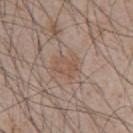Acquisition and patient details:
Located on the chest. Approximately 3 mm at its widest. Cropped from a total-body skin-imaging series; the visible field is about 15 mm. A male subject, roughly 45 years of age. The total-body-photography lesion software estimated an average lesion color of about L≈52 a*≈17 b*≈27 (CIELAB), about 6 CIELAB-L* units darker than the surrounding skin, and a lesion-to-skin contrast of about 5 (normalized; higher = more distinct). The tile uses white-light illumination.